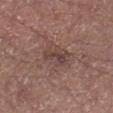anatomic site: the leg | subject: male, in their mid- to late 50s | acquisition: total-body-photography crop, ~15 mm field of view.